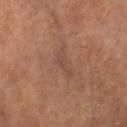Q: Is there a histopathology result?
A: total-body-photography surveillance lesion; no biopsy
Q: Lesion location?
A: the right thigh
Q: What is the imaging modality?
A: ~15 mm tile from a whole-body skin photo
Q: How was the tile lit?
A: cross-polarized
Q: Patient demographics?
A: male, aged 83 or over
Q: What is the lesion's diameter?
A: about 3.5 mm
Q: What did automated image analysis measure?
A: a footprint of about 4 mm², an eccentricity of roughly 0.9, and two-axis asymmetry of about 0.55; an average lesion color of about L≈45 a*≈20 b*≈25 (CIELAB) and roughly 5 lightness units darker than nearby skin; a color-variation rating of about 1/10 and radial color variation of about 0.5